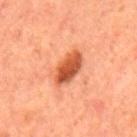follow-up: no biopsy performed (imaged during a skin exam) | lighting: cross-polarized | lesion diameter: about 4.5 mm | subject: male, aged around 65 | anatomic site: the mid back | image: ~15 mm tile from a whole-body skin photo | image-analysis metrics: an area of roughly 8 mm², a shape eccentricity near 0.9, and two-axis asymmetry of about 0.15; a mean CIELAB color near L≈49 a*≈31 b*≈38, roughly 15 lightness units darker than nearby skin, and a lesion-to-skin contrast of about 10.5 (normalized; higher = more distinct); a detector confidence of about 100 out of 100 that the crop contains a lesion.